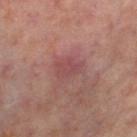{
  "biopsy_status": "not biopsied; imaged during a skin examination",
  "site": "left thigh",
  "lighting": "cross-polarized",
  "image": {
    "source": "total-body photography crop",
    "field_of_view_mm": 15
  },
  "patient": {
    "sex": "female",
    "age_approx": 50
  },
  "lesion_size": {
    "long_diameter_mm_approx": 3.0
  }
}